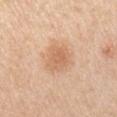No biopsy was performed on this lesion — it was imaged during a full skin examination and was not determined to be concerning. This is a white-light tile. From the left upper arm. A lesion tile, about 15 mm wide, cut from a 3D total-body photograph. An algorithmic analysis of the crop reported an area of roughly 8 mm² and a shape eccentricity near 0.55. The software also gave an automated nevus-likeness rating near 30 out of 100 and a detector confidence of about 100 out of 100 that the crop contains a lesion. A male subject, approximately 60 years of age. Measured at roughly 3.5 mm in maximum diameter.